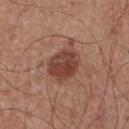<tbp_lesion>
  <biopsy_status>not biopsied; imaged during a skin examination</biopsy_status>
  <site>chest</site>
  <image>
    <source>total-body photography crop</source>
    <field_of_view_mm>15</field_of_view_mm>
  </image>
  <lighting>white-light</lighting>
  <lesion_size>
    <long_diameter_mm_approx>5.5</long_diameter_mm_approx>
  </lesion_size>
  <automated_metrics>
    <cielab_L>43</cielab_L>
    <cielab_a>23</cielab_a>
    <cielab_b>27</cielab_b>
    <vs_skin_contrast_norm>9.0</vs_skin_contrast_norm>
    <nevus_likeness_0_100>90</nevus_likeness_0_100>
  </automated_metrics>
  <patient>
    <sex>male</sex>
    <age_approx>55</age_approx>
  </patient>
</tbp_lesion>